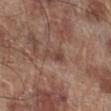Impression:
Recorded during total-body skin imaging; not selected for excision or biopsy.
Acquisition and patient details:
This image is a 15 mm lesion crop taken from a total-body photograph. Captured under white-light illumination. The subject is a male aged approximately 70. The total-body-photography lesion software estimated an average lesion color of about L≈44 a*≈19 b*≈25 (CIELAB), about 8 CIELAB-L* units darker than the surrounding skin, and a normalized lesion–skin contrast near 6.5. About 2.5 mm across. Located on the right lower leg.